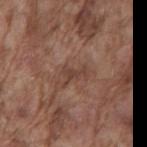Assessment:
No biopsy was performed on this lesion — it was imaged during a full skin examination and was not determined to be concerning.